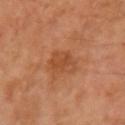workup — total-body-photography surveillance lesion; no biopsy | illumination — cross-polarized illumination | subject — female, aged around 55 | anatomic site — the right upper arm | acquisition — ~15 mm crop, total-body skin-cancer survey | automated metrics — an area of roughly 7 mm², a shape eccentricity near 0.8, and a symmetry-axis asymmetry near 0.25; about 7 CIELAB-L* units darker than the surrounding skin and a normalized border contrast of about 6.